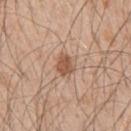Case summary:
• biopsy status · imaged on a skin check; not biopsied
• diameter · ~2.5 mm (longest diameter)
• automated metrics · an outline eccentricity of about 0.6 (0 = round, 1 = elongated) and two-axis asymmetry of about 0.2; a lesion color around L≈54 a*≈20 b*≈31 in CIELAB, a lesion–skin lightness drop of about 11, and a lesion-to-skin contrast of about 8 (normalized; higher = more distinct)
• patient · male, approximately 50 years of age
• image source · 15 mm crop, total-body photography
• anatomic site · the right upper arm